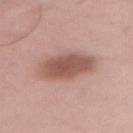No biopsy was performed on this lesion — it was imaged during a full skin examination and was not determined to be concerning. A 15 mm close-up extracted from a 3D total-body photography capture. The lesion is on the abdomen. The subject is a male about 55 years old.